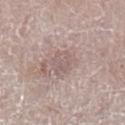Q: Was a biopsy performed?
A: no biopsy performed (imaged during a skin exam)
Q: What is the anatomic site?
A: the leg
Q: What are the patient's age and sex?
A: female, about 70 years old
Q: How large is the lesion?
A: about 3 mm
Q: How was this image acquired?
A: ~15 mm tile from a whole-body skin photo
Q: How was the tile lit?
A: white-light illumination